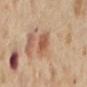Case summary:
- biopsy status: total-body-photography surveillance lesion; no biopsy
- anatomic site: the lower back
- imaging modality: 15 mm crop, total-body photography
- tile lighting: cross-polarized illumination
- automated metrics: an outline eccentricity of about 0.65 (0 = round, 1 = elongated) and a shape-asymmetry score of about 0.25 (0 = symmetric); about 10 CIELAB-L* units darker than the surrounding skin and a lesion-to-skin contrast of about 7 (normalized; higher = more distinct); border irregularity of about 2.5 on a 0–10 scale and radial color variation of about 1
- patient: female, aged around 55
- size: about 3 mm